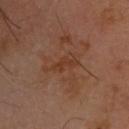biopsy status = no biopsy performed (imaged during a skin exam) | subject = male, aged around 70 | site = the head or neck | imaging modality = 15 mm crop, total-body photography | illumination = cross-polarized illumination | diameter = about 3.5 mm.